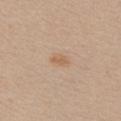<lesion>
<biopsy_status>not biopsied; imaged during a skin examination</biopsy_status>
<lesion_size>
  <long_diameter_mm_approx>2.5</long_diameter_mm_approx>
</lesion_size>
<image>
  <source>total-body photography crop</source>
  <field_of_view_mm>15</field_of_view_mm>
</image>
<lighting>white-light</lighting>
<site>chest</site>
<patient>
  <sex>female</sex>
  <age_approx>35</age_approx>
</patient>
</lesion>